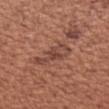notes: total-body-photography surveillance lesion; no biopsy | patient: female, aged 48–52 | body site: the left upper arm | lesion size: ≈5 mm | image: ~15 mm crop, total-body skin-cancer survey | tile lighting: white-light.